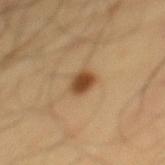Part of a total-body skin-imaging series; this lesion was reviewed on a skin check and was not flagged for biopsy. The lesion is located on the mid back. Approximately 2.5 mm at its widest. A male patient, aged around 55. Automated tile analysis of the lesion measured an automated nevus-likeness rating near 100 out of 100 and a lesion-detection confidence of about 100/100. A 15 mm crop from a total-body photograph taken for skin-cancer surveillance.